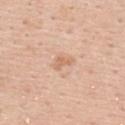Assessment:
Imaged during a routine full-body skin examination; the lesion was not biopsied and no histopathology is available.
Clinical summary:
About 2.5 mm across. The tile uses white-light illumination. Located on the upper back. The patient is a female in their mid-40s. A 15 mm close-up tile from a total-body photography series done for melanoma screening. The total-body-photography lesion software estimated an area of roughly 3 mm², an eccentricity of roughly 0.8, and a symmetry-axis asymmetry near 0.5. It also reported a lesion color around L≈66 a*≈21 b*≈33 in CIELAB, roughly 8 lightness units darker than nearby skin, and a normalized border contrast of about 5.5. The analysis additionally found a border-irregularity rating of about 4.5/10, a color-variation rating of about 0.5/10, and a peripheral color-asymmetry measure near 0. It also reported a classifier nevus-likeness of about 0/100 and lesion-presence confidence of about 100/100.